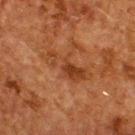follow-up — total-body-photography surveillance lesion; no biopsy | acquisition — ~15 mm tile from a whole-body skin photo | location — the back | patient — male, aged approximately 60.